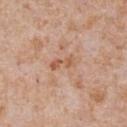notes: no biopsy performed (imaged during a skin exam)
illumination: white-light
subject: male, aged approximately 65
imaging modality: ~15 mm crop, total-body skin-cancer survey
automated metrics: an area of roughly 4 mm², a shape eccentricity near 0.9, and two-axis asymmetry of about 0.35; a lesion–skin lightness drop of about 8 and a normalized lesion–skin contrast near 6.5; a border-irregularity rating of about 5/10, internal color variation of about 0.5 on a 0–10 scale, and radial color variation of about 0; a classifier nevus-likeness of about 0/100 and a detector confidence of about 100 out of 100 that the crop contains a lesion
anatomic site: the chest
size: ≈3 mm